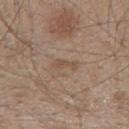The patient is a male in their mid-40s. This is a white-light tile. Approximately 3 mm at its widest. Located on the mid back. Cropped from a whole-body photographic skin survey; the tile spans about 15 mm.